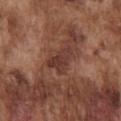follow-up — total-body-photography surveillance lesion; no biopsy
image source — 15 mm crop, total-body photography
lesion diameter — ~3.5 mm (longest diameter)
subject — male, approximately 75 years of age
location — the chest
illumination — white-light